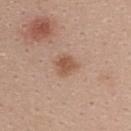Q: Is there a histopathology result?
A: total-body-photography surveillance lesion; no biopsy
Q: What is the lesion's diameter?
A: ~2.5 mm (longest diameter)
Q: What is the imaging modality?
A: 15 mm crop, total-body photography
Q: What did automated image analysis measure?
A: border irregularity of about 2.5 on a 0–10 scale, a color-variation rating of about 2.5/10, and peripheral color asymmetry of about 1; a detector confidence of about 100 out of 100 that the crop contains a lesion
Q: What are the patient's age and sex?
A: female, aged approximately 25
Q: Where on the body is the lesion?
A: the upper back